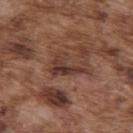Assessment:
No biopsy was performed on this lesion — it was imaged during a full skin examination and was not determined to be concerning.